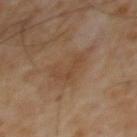Impression:
Captured during whole-body skin photography for melanoma surveillance; the lesion was not biopsied.
Background:
A 15 mm close-up extracted from a 3D total-body photography capture. Imaged with cross-polarized lighting. The subject is a male aged 63 to 67. About 4.5 mm across.